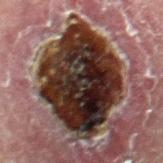– notes · catalogued during a skin exam; not biopsied
– location · the right thigh
– image source · ~15 mm crop, total-body skin-cancer survey
– subject · male, in their 60s